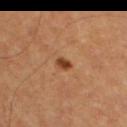| key | value |
|---|---|
| follow-up | total-body-photography surveillance lesion; no biopsy |